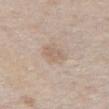• imaging modality — total-body-photography crop, ~15 mm field of view
• tile lighting — white-light illumination
• subject — male, in their mid-70s
• body site — the front of the torso
• lesion diameter — ≈2.5 mm